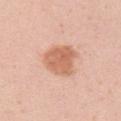follow-up: no biopsy performed (imaged during a skin exam) | lesion size: ~4 mm (longest diameter) | subject: female, roughly 30 years of age | automated lesion analysis: a classifier nevus-likeness of about 70/100 and lesion-presence confidence of about 100/100 | lighting: white-light | imaging modality: ~15 mm tile from a whole-body skin photo | body site: the right upper arm.